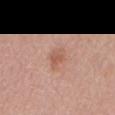Q: Is there a histopathology result?
A: no biopsy performed (imaged during a skin exam)
Q: Lesion location?
A: the mid back
Q: Illumination type?
A: white-light illumination
Q: What did automated image analysis measure?
A: an area of roughly 4.5 mm² and a symmetry-axis asymmetry near 0.3; a mean CIELAB color near L≈57 a*≈22 b*≈30 and about 8 CIELAB-L* units darker than the surrounding skin
Q: How was this image acquired?
A: ~15 mm tile from a whole-body skin photo
Q: Who is the patient?
A: male, aged 63 to 67